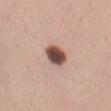No biopsy was performed on this lesion — it was imaged during a full skin examination and was not determined to be concerning. An algorithmic analysis of the crop reported a nevus-likeness score of about 100/100 and a lesion-detection confidence of about 100/100. Longest diameter approximately 3 mm. The tile uses white-light illumination. A region of skin cropped from a whole-body photographic capture, roughly 15 mm wide. Located on the front of the torso. A female patient, approximately 35 years of age.